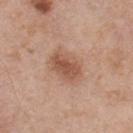Assessment: Imaged during a routine full-body skin examination; the lesion was not biopsied and no histopathology is available. Context: Captured under white-light illumination. Approximately 4 mm at its widest. Automated image analysis of the tile measured a lesion area of about 10 mm², an outline eccentricity of about 0.75 (0 = round, 1 = elongated), and a symmetry-axis asymmetry near 0.1. The patient is a male approximately 55 years of age. The lesion is on the back. A 15 mm close-up tile from a total-body photography series done for melanoma screening.